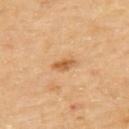No biopsy was performed on this lesion — it was imaged during a full skin examination and was not determined to be concerning.
This is a cross-polarized tile.
Located on the back.
A female patient, aged approximately 70.
A lesion tile, about 15 mm wide, cut from a 3D total-body photograph.
Longest diameter approximately 2.5 mm.